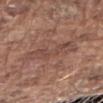workup: imaged on a skin check; not biopsied
location: the right forearm
patient: male, aged 73 to 77
image-analysis metrics: a lesion area of about 9.5 mm², an eccentricity of roughly 0.95, and two-axis asymmetry of about 0.3; a color-variation rating of about 3.5/10 and radial color variation of about 1; a nevus-likeness score of about 0/100 and a detector confidence of about 75 out of 100 that the crop contains a lesion
size: ≈5.5 mm
tile lighting: white-light illumination
image source: ~15 mm crop, total-body skin-cancer survey